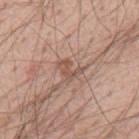biopsy status = total-body-photography surveillance lesion; no biopsy
acquisition = ~15 mm crop, total-body skin-cancer survey
site = the mid back
patient = male, aged approximately 55